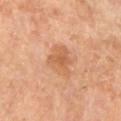<case>
<biopsy_status>not biopsied; imaged during a skin examination</biopsy_status>
<patient>
  <sex>female</sex>
  <age_approx>70</age_approx>
</patient>
<lighting>cross-polarized</lighting>
<lesion_size>
  <long_diameter_mm_approx>4.0</long_diameter_mm_approx>
</lesion_size>
<image>
  <source>total-body photography crop</source>
  <field_of_view_mm>15</field_of_view_mm>
</image>
<site>leg</site>
</case>